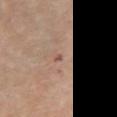Findings:
– workup: imaged on a skin check; not biopsied
– body site: the chest
– patient: female, aged around 65
– imaging modality: ~15 mm crop, total-body skin-cancer survey
– lesion diameter: about 1 mm
– illumination: white-light